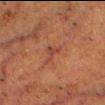A lesion tile, about 15 mm wide, cut from a 3D total-body photograph. Automated image analysis of the tile measured a border-irregularity index near 6.5/10, a within-lesion color-variation index near 1/10, and a peripheral color-asymmetry measure near 0.5. The analysis additionally found a lesion-detection confidence of about 70/100. A male patient, about 50 years old. Imaged with cross-polarized lighting. From the head or neck.